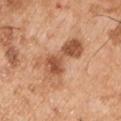Captured during whole-body skin photography for melanoma surveillance; the lesion was not biopsied. Imaged with white-light lighting. Automated image analysis of the tile measured an area of roughly 16 mm², an eccentricity of roughly 0.9, and a shape-asymmetry score of about 0.55 (0 = symmetric). The software also gave a border-irregularity rating of about 8.5/10, a color-variation rating of about 5.5/10, and peripheral color asymmetry of about 2. The analysis additionally found an automated nevus-likeness rating near 0 out of 100 and lesion-presence confidence of about 100/100. A male patient, about 55 years old. The lesion's longest dimension is about 7 mm. From the left upper arm. A close-up tile cropped from a whole-body skin photograph, about 15 mm across.